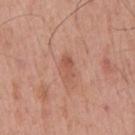Recorded during total-body skin imaging; not selected for excision or biopsy.
About 3 mm across.
Captured under white-light illumination.
From the mid back.
Cropped from a total-body skin-imaging series; the visible field is about 15 mm.
An algorithmic analysis of the crop reported a detector confidence of about 100 out of 100 that the crop contains a lesion.
The patient is a male aged around 55.